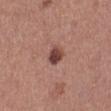Clinical impression:
Part of a total-body skin-imaging series; this lesion was reviewed on a skin check and was not flagged for biopsy.
Background:
On the left lower leg. A 15 mm close-up extracted from a 3D total-body photography capture. A female patient aged around 60.